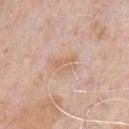site: the chest
illumination: white-light
lesion diameter: about 3 mm
imaging modality: ~15 mm crop, total-body skin-cancer survey
subject: male, aged approximately 70